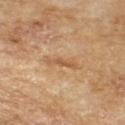No biopsy was performed on this lesion — it was imaged during a full skin examination and was not determined to be concerning. The recorded lesion diameter is about 3.5 mm. A 15 mm close-up extracted from a 3D total-body photography capture. A male patient, aged approximately 65. Imaged with cross-polarized lighting.